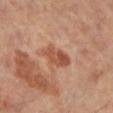Findings:
– biopsy status · catalogued during a skin exam; not biopsied
– diameter · ≈4 mm
– subject · female, aged 58–62
– body site · the left lower leg
– automated metrics · a footprint of about 7 mm², a shape eccentricity near 0.8, and two-axis asymmetry of about 0.25; a mean CIELAB color near L≈53 a*≈26 b*≈34 and roughly 11 lightness units darker than nearby skin; border irregularity of about 2.5 on a 0–10 scale and a within-lesion color-variation index near 4.5/10
– image · ~15 mm tile from a whole-body skin photo